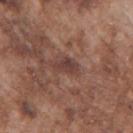Findings:
• workup — no biopsy performed (imaged during a skin exam)
• location — the right upper arm
• tile lighting — white-light
• image — 15 mm crop, total-body photography
• image-analysis metrics — a lesion area of about 4.5 mm², a shape eccentricity near 0.8, and two-axis asymmetry of about 0.25; a color-variation rating of about 2.5/10 and peripheral color asymmetry of about 1; a classifier nevus-likeness of about 0/100 and a lesion-detection confidence of about 90/100
• diameter — about 3 mm
• subject — male, about 75 years old